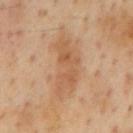Q: Is there a histopathology result?
A: total-body-photography surveillance lesion; no biopsy
Q: What are the patient's age and sex?
A: male, aged approximately 55
Q: What is the imaging modality?
A: total-body-photography crop, ~15 mm field of view
Q: What lighting was used for the tile?
A: cross-polarized illumination
Q: What is the anatomic site?
A: the mid back